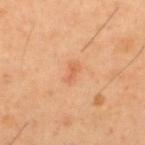Q: Is there a histopathology result?
A: no biopsy performed (imaged during a skin exam)
Q: Lesion size?
A: ~2.5 mm (longest diameter)
Q: How was this image acquired?
A: ~15 mm tile from a whole-body skin photo
Q: Patient demographics?
A: male, aged 38–42
Q: What is the anatomic site?
A: the upper back
Q: What lighting was used for the tile?
A: cross-polarized